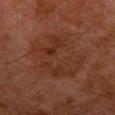Recorded during total-body skin imaging; not selected for excision or biopsy. Imaged with cross-polarized lighting. On the leg. A male patient in their 80s. Cropped from a total-body skin-imaging series; the visible field is about 15 mm. Automated image analysis of the tile measured a lesion color around L≈25 a*≈17 b*≈23 in CIELAB and a normalized lesion–skin contrast near 5. The recorded lesion diameter is about 8 mm.